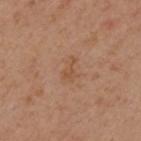<record>
  <biopsy_status>not biopsied; imaged during a skin examination</biopsy_status>
  <patient>
    <sex>male</sex>
    <age_approx>45</age_approx>
  </patient>
  <site>upper back</site>
  <image>
    <source>total-body photography crop</source>
    <field_of_view_mm>15</field_of_view_mm>
  </image>
</record>